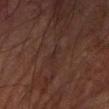– follow-up — catalogued during a skin exam; not biopsied
– diameter — ~2 mm (longest diameter)
– site — the right forearm
– patient — male, approximately 65 years of age
– lighting — cross-polarized
– image — total-body-photography crop, ~15 mm field of view